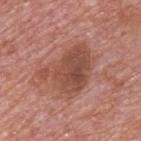Recorded during total-body skin imaging; not selected for excision or biopsy. The lesion is located on the upper back. A male subject, aged approximately 75. Cropped from a total-body skin-imaging series; the visible field is about 15 mm.